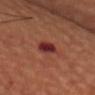biopsy status: no biopsy performed (imaged during a skin exam) | site: the chest | subject: female, approximately 45 years of age | image source: ~15 mm tile from a whole-body skin photo.